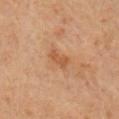<tbp_lesion>
  <biopsy_status>not biopsied; imaged during a skin examination</biopsy_status>
  <image>
    <source>total-body photography crop</source>
    <field_of_view_mm>15</field_of_view_mm>
  </image>
  <automated_metrics>
    <eccentricity>0.85</eccentricity>
    <shape_asymmetry>0.25</shape_asymmetry>
    <vs_skin_darker_L>7.0</vs_skin_darker_L>
    <vs_skin_contrast_norm>6.0</vs_skin_contrast_norm>
  </automated_metrics>
  <patient>
    <sex>male</sex>
    <age_approx>60</age_approx>
  </patient>
  <site>right upper arm</site>
  <lesion_size>
    <long_diameter_mm_approx>3.0</long_diameter_mm_approx>
  </lesion_size>
</tbp_lesion>